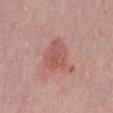The lesion was photographed on a routine skin check and not biopsied; there is no pathology result. On the mid back. A 15 mm crop from a total-body photograph taken for skin-cancer surveillance. A female patient in their mid- to late 60s.